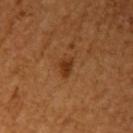  biopsy_status: not biopsied; imaged during a skin examination
  image:
    source: total-body photography crop
    field_of_view_mm: 15
  lighting: cross-polarized
  site: left upper arm
  automated_metrics:
    area_mm2_approx: 4.0
    eccentricity: 0.7
    cielab_L: 36
    cielab_a: 24
    cielab_b: 37
    vs_skin_darker_L: 9.0
    vs_skin_contrast_norm: 7.5
    color_variation_0_10: 2.5
    peripheral_color_asymmetry: 0.5
  lesion_size:
    long_diameter_mm_approx: 3.0
  patient:
    sex: female
    age_approx: 55